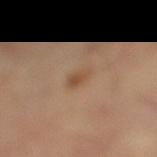workup=imaged on a skin check; not biopsied | illumination=cross-polarized illumination | site=the leg | lesion size=~2.5 mm (longest diameter) | image=~15 mm tile from a whole-body skin photo | subject=female, about 60 years old.